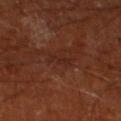Part of a total-body skin-imaging series; this lesion was reviewed on a skin check and was not flagged for biopsy.